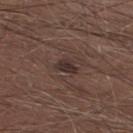<lesion>
  <site>right lower leg</site>
  <image>
    <source>total-body photography crop</source>
    <field_of_view_mm>15</field_of_view_mm>
  </image>
  <patient>
    <sex>male</sex>
    <age_approx>25</age_approx>
  </patient>
</lesion>